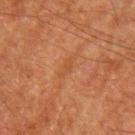Q: Is there a histopathology result?
A: total-body-photography surveillance lesion; no biopsy
Q: How large is the lesion?
A: ≈3 mm
Q: What kind of image is this?
A: ~15 mm crop, total-body skin-cancer survey
Q: Who is the patient?
A: male, roughly 80 years of age
Q: Automated lesion metrics?
A: a nevus-likeness score of about 0/100 and a detector confidence of about 100 out of 100 that the crop contains a lesion
Q: Where on the body is the lesion?
A: the left upper arm